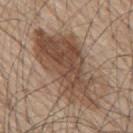workup = imaged on a skin check; not biopsied
patient = male, approximately 70 years of age
body site = the back
lighting = white-light illumination
imaging modality = 15 mm crop, total-body photography
lesion diameter = ~11.5 mm (longest diameter)